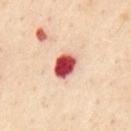biopsy status: no biopsy performed (imaged during a skin exam) | patient: male, aged approximately 55 | diameter: ~3.5 mm (longest diameter) | TBP lesion metrics: a footprint of about 7 mm², an outline eccentricity of about 0.6 (0 = round, 1 = elongated), and a symmetry-axis asymmetry near 0.15 | lighting: cross-polarized illumination | body site: the chest | acquisition: 15 mm crop, total-body photography.